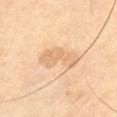Q: Is there a histopathology result?
A: no biopsy performed (imaged during a skin exam)
Q: What is the imaging modality?
A: ~15 mm tile from a whole-body skin photo
Q: Lesion location?
A: the left thigh
Q: What lighting was used for the tile?
A: cross-polarized
Q: Lesion size?
A: about 5 mm
Q: Patient demographics?
A: male, aged around 65